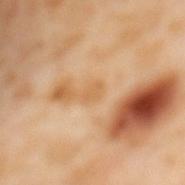Impression: No biopsy was performed on this lesion — it was imaged during a full skin examination and was not determined to be concerning. Image and clinical context: On the mid back. A female subject about 55 years old. Cropped from a whole-body photographic skin survey; the tile spans about 15 mm.